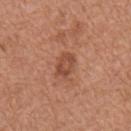Assessment: The lesion was tiled from a total-body skin photograph and was not biopsied. Clinical summary: Approximately 3 mm at its widest. The lesion-visualizer software estimated a shape eccentricity near 0.8 and a symmetry-axis asymmetry near 0.2. It also reported an automated nevus-likeness rating near 45 out of 100. This is a white-light tile. From the mid back. The subject is a male in their mid-60s. A lesion tile, about 15 mm wide, cut from a 3D total-body photograph.